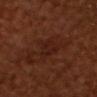Context:
The lesion is located on the head or neck. A male patient, aged 63 to 67. The lesion-visualizer software estimated an area of roughly 4.5 mm², an eccentricity of roughly 0.7, and a shape-asymmetry score of about 0.25 (0 = symmetric). It also reported an average lesion color of about L≈20 a*≈21 b*≈23 (CIELAB) and a lesion–skin lightness drop of about 4. Cropped from a whole-body photographic skin survey; the tile spans about 15 mm.